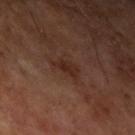Captured during whole-body skin photography for melanoma surveillance; the lesion was not biopsied. Imaged with cross-polarized lighting. A lesion tile, about 15 mm wide, cut from a 3D total-body photograph. From the left upper arm. A male subject aged 63 to 67.